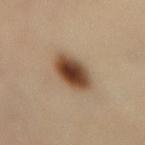Clinical impression:
The lesion was tiled from a total-body skin photograph and was not biopsied.
Clinical summary:
On the lower back. A female patient, aged approximately 50. An algorithmic analysis of the crop reported a footprint of about 11 mm², an outline eccentricity of about 0.65 (0 = round, 1 = elongated), and a symmetry-axis asymmetry near 0.1. And it measured an average lesion color of about L≈45 a*≈19 b*≈31 (CIELAB), about 18 CIELAB-L* units darker than the surrounding skin, and a normalized lesion–skin contrast near 13. The software also gave a classifier nevus-likeness of about 100/100. The tile uses cross-polarized illumination. A 15 mm close-up tile from a total-body photography series done for melanoma screening.